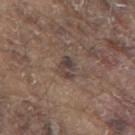Q: Lesion size?
A: about 2.5 mm
Q: Who is the patient?
A: male, aged 78 to 82
Q: What lighting was used for the tile?
A: white-light illumination
Q: Lesion location?
A: the mid back
Q: What is the imaging modality?
A: 15 mm crop, total-body photography